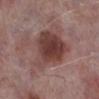The lesion was tiled from a total-body skin photograph and was not biopsied.
This image is a 15 mm lesion crop taken from a total-body photograph.
The patient is a male aged 73–77.
The recorded lesion diameter is about 8.5 mm.
On the right lower leg.
The total-body-photography lesion software estimated a footprint of about 30 mm² and two-axis asymmetry of about 0.3. The analysis additionally found a mean CIELAB color near L≈42 a*≈20 b*≈21 and a normalized lesion–skin contrast near 9. It also reported peripheral color asymmetry of about 2.
This is a white-light tile.